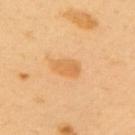The lesion was photographed on a routine skin check and not biopsied; there is no pathology result.
A female patient roughly 50 years of age.
A lesion tile, about 15 mm wide, cut from a 3D total-body photograph.
Automated image analysis of the tile measured a footprint of about 5.5 mm² and two-axis asymmetry of about 0.2. And it measured an average lesion color of about L≈65 a*≈22 b*≈45 (CIELAB), roughly 8 lightness units darker than nearby skin, and a lesion-to-skin contrast of about 6 (normalized; higher = more distinct). The software also gave a within-lesion color-variation index near 2.5/10. And it measured a nevus-likeness score of about 30/100.
Imaged with cross-polarized lighting.
Approximately 3.5 mm at its widest.
The lesion is located on the upper back.